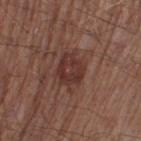Imaged during a routine full-body skin examination; the lesion was not biopsied and no histopathology is available. A male patient aged 53 to 57. Cropped from a whole-body photographic skin survey; the tile spans about 15 mm. The lesion is on the left thigh.